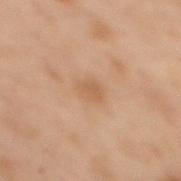notes = total-body-photography surveillance lesion; no biopsy
TBP lesion metrics = a footprint of about 4 mm²; an average lesion color of about L≈49 a*≈16 b*≈30 (CIELAB); a nevus-likeness score of about 5/100 and lesion-presence confidence of about 100/100
site = the upper back
subject = female, aged 53 to 57
size = about 2.5 mm
illumination = cross-polarized illumination
imaging modality = ~15 mm crop, total-body skin-cancer survey